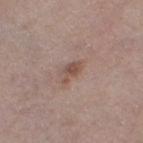Impression: The lesion was photographed on a routine skin check and not biopsied; there is no pathology result. Background: Cropped from a total-body skin-imaging series; the visible field is about 15 mm. Automated image analysis of the tile measured a lesion color around L≈50 a*≈18 b*≈24 in CIELAB, about 9 CIELAB-L* units darker than the surrounding skin, and a lesion-to-skin contrast of about 7 (normalized; higher = more distinct). The software also gave a border-irregularity rating of about 4.5/10, internal color variation of about 1.5 on a 0–10 scale, and peripheral color asymmetry of about 0.5. A female patient, about 60 years old. Approximately 3 mm at its widest. Captured under white-light illumination. The lesion is located on the left thigh.